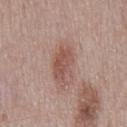biopsy_status: not biopsied; imaged during a skin examination
image:
  source: total-body photography crop
  field_of_view_mm: 15
patient:
  sex: male
  age_approx: 70
automated_metrics:
  area_mm2_approx: 8.5
  eccentricity: 0.85
  shape_asymmetry: 0.25
  color_variation_0_10: 3.0
lesion_size:
  long_diameter_mm_approx: 4.5
site: mid back
lighting: white-light